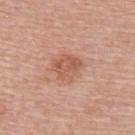Recorded during total-body skin imaging; not selected for excision or biopsy. The total-body-photography lesion software estimated a footprint of about 8.5 mm², an outline eccentricity of about 0.55 (0 = round, 1 = elongated), and a shape-asymmetry score of about 0.25 (0 = symmetric). And it measured an automated nevus-likeness rating near 20 out of 100 and a detector confidence of about 100 out of 100 that the crop contains a lesion. The recorded lesion diameter is about 3.5 mm. The patient is a female roughly 50 years of age. The tile uses white-light illumination. Cropped from a whole-body photographic skin survey; the tile spans about 15 mm. Located on the back.